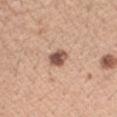acquisition: total-body-photography crop, ~15 mm field of view
subject: female, about 45 years old
body site: the left forearm
lesion diameter: ~3 mm (longest diameter)